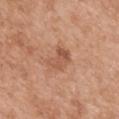* workup: total-body-photography surveillance lesion; no biopsy
* automated lesion analysis: a footprint of about 6 mm² and an outline eccentricity of about 0.65 (0 = round, 1 = elongated); a border-irregularity rating of about 3.5/10, internal color variation of about 3 on a 0–10 scale, and radial color variation of about 1; a classifier nevus-likeness of about 5/100
* tile lighting: white-light illumination
* anatomic site: the mid back
* imaging modality: total-body-photography crop, ~15 mm field of view
* size: ~3 mm (longest diameter)
* subject: female, about 40 years old